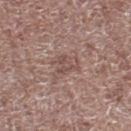The subject is a male aged 68 to 72.
Cropped from a whole-body photographic skin survey; the tile spans about 15 mm.
About 3 mm across.
The tile uses white-light illumination.
The lesion is located on the left lower leg.